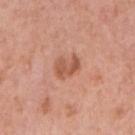From the right upper arm. Cropped from a total-body skin-imaging series; the visible field is about 15 mm. The total-body-photography lesion software estimated a color-variation rating of about 3/10. The patient is a female approximately 60 years of age.